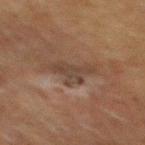A male subject, roughly 75 years of age. A roughly 15 mm field-of-view crop from a total-body skin photograph. Measured at roughly 4.5 mm in maximum diameter. Captured under cross-polarized illumination. Located on the back.